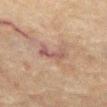Notes:
- workup · no biopsy performed (imaged during a skin exam)
- body site · the abdomen
- subject · male, aged around 75
- tile lighting · cross-polarized illumination
- size · about 4 mm
- imaging modality · ~15 mm tile from a whole-body skin photo